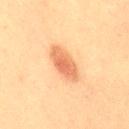image:
  source: total-body photography crop
  field_of_view_mm: 15
lesion_size:
  long_diameter_mm_approx: 5.0
patient:
  sex: female
  age_approx: 50
automated_metrics:
  cielab_L: 59
  cielab_a: 24
  cielab_b: 36
  vs_skin_darker_L: 11.0
  border_irregularity_0_10: 2.0
  color_variation_0_10: 4.0
  peripheral_color_asymmetry: 1.0
site: right thigh